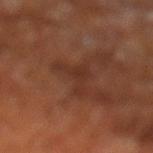Clinical impression:
Recorded during total-body skin imaging; not selected for excision or biopsy.
Background:
The lesion's longest dimension is about 4 mm. The total-body-photography lesion software estimated a border-irregularity rating of about 8/10 and a color-variation rating of about 1.5/10. The analysis additionally found a detector confidence of about 100 out of 100 that the crop contains a lesion. On the left lower leg. Cropped from a whole-body photographic skin survey; the tile spans about 15 mm. The patient is a male approximately 65 years of age.